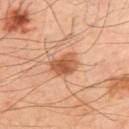Part of a total-body skin-imaging series; this lesion was reviewed on a skin check and was not flagged for biopsy. The lesion is located on the upper back. The tile uses cross-polarized illumination. Cropped from a total-body skin-imaging series; the visible field is about 15 mm. A male subject, aged approximately 50.